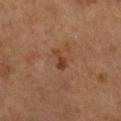The lesion was tiled from a total-body skin photograph and was not biopsied.
A female subject, aged around 65.
The lesion is located on the right lower leg.
A 15 mm close-up extracted from a 3D total-body photography capture.
The lesion's longest dimension is about 3 mm.